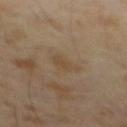Captured during whole-body skin photography for melanoma surveillance; the lesion was not biopsied. Cropped from a total-body skin-imaging series; the visible field is about 15 mm. The tile uses cross-polarized illumination. Measured at roughly 2.5 mm in maximum diameter. The subject is a male roughly 65 years of age. Automated image analysis of the tile measured a lesion area of about 3.5 mm², an eccentricity of roughly 0.8, and a symmetry-axis asymmetry near 0.35. It also reported an average lesion color of about L≈44 a*≈13 b*≈29 (CIELAB), roughly 5 lightness units darker than nearby skin, and a normalized lesion–skin contrast near 5. The software also gave a border-irregularity index near 4/10, internal color variation of about 1.5 on a 0–10 scale, and a peripheral color-asymmetry measure near 0.5.